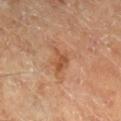Case summary:
* biopsy status · no biopsy performed (imaged during a skin exam)